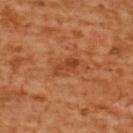Assessment:
The lesion was tiled from a total-body skin photograph and was not biopsied.
Acquisition and patient details:
The lesion-visualizer software estimated an area of roughly 4.5 mm², an outline eccentricity of about 0.9 (0 = round, 1 = elongated), and a shape-asymmetry score of about 0.2 (0 = symmetric). And it measured an average lesion color of about L≈46 a*≈29 b*≈39 (CIELAB) and a normalized border contrast of about 6.5. Imaged with cross-polarized lighting. Located on the upper back. A region of skin cropped from a whole-body photographic capture, roughly 15 mm wide. The lesion's longest dimension is about 3.5 mm. A female patient, roughly 55 years of age.